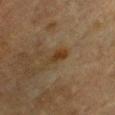Findings:
* notes: catalogued during a skin exam; not biopsied
* location: the chest
* lighting: cross-polarized
* diameter: ≈3 mm
* patient: male, roughly 85 years of age or older
* image: 15 mm crop, total-body photography